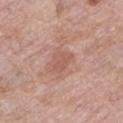Captured during whole-body skin photography for melanoma surveillance; the lesion was not biopsied.
On the left lower leg.
Automated tile analysis of the lesion measured a lesion area of about 4.5 mm² and an outline eccentricity of about 0.7 (0 = round, 1 = elongated). It also reported a mean CIELAB color near L≈56 a*≈23 b*≈28 and about 7 CIELAB-L* units darker than the surrounding skin. It also reported a border-irregularity rating of about 3/10, internal color variation of about 1.5 on a 0–10 scale, and peripheral color asymmetry of about 0.5.
A female patient in their 70s.
A 15 mm close-up extracted from a 3D total-body photography capture.
This is a white-light tile.